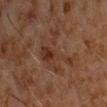Imaged during a routine full-body skin examination; the lesion was not biopsied and no histopathology is available.
This is a cross-polarized tile.
The lesion is on the chest.
Automated image analysis of the tile measured a mean CIELAB color near L≈32 a*≈19 b*≈26, a lesion–skin lightness drop of about 6, and a lesion-to-skin contrast of about 6 (normalized; higher = more distinct). It also reported a color-variation rating of about 6/10 and a peripheral color-asymmetry measure near 2.5. The analysis additionally found an automated nevus-likeness rating near 0 out of 100.
Approximately 6 mm at its widest.
A region of skin cropped from a whole-body photographic capture, roughly 15 mm wide.
The subject is a male approximately 60 years of age.